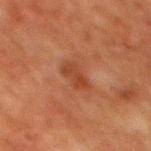Cropped from a whole-body photographic skin survey; the tile spans about 15 mm. The lesion is located on the mid back. The lesion-visualizer software estimated an area of roughly 5 mm² and a shape-asymmetry score of about 0.25 (0 = symmetric). The analysis additionally found a mean CIELAB color near L≈34 a*≈23 b*≈29, a lesion–skin lightness drop of about 7, and a lesion-to-skin contrast of about 6.5 (normalized; higher = more distinct). And it measured a border-irregularity rating of about 2.5/10, a within-lesion color-variation index near 2.5/10, and peripheral color asymmetry of about 1. The software also gave an automated nevus-likeness rating near 0 out of 100 and lesion-presence confidence of about 100/100. About 3.5 mm across. The subject is a male approximately 80 years of age. Captured under cross-polarized illumination.